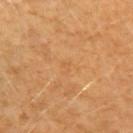biopsy status — catalogued during a skin exam; not biopsied
size — ≈1 mm
image source — 15 mm crop, total-body photography
location — the left upper arm
subject — female, aged around 50